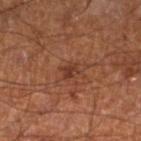Part of a total-body skin-imaging series; this lesion was reviewed on a skin check and was not flagged for biopsy.
A 15 mm close-up tile from a total-body photography series done for melanoma screening.
Automated image analysis of the tile measured a footprint of about 3 mm², a shape eccentricity near 0.7, and a symmetry-axis asymmetry near 0.25. The software also gave border irregularity of about 2.5 on a 0–10 scale, internal color variation of about 1 on a 0–10 scale, and a peripheral color-asymmetry measure near 0.5. The analysis additionally found a classifier nevus-likeness of about 20/100 and a lesion-detection confidence of about 100/100.
The lesion is located on the left lower leg.
A male patient in their 60s.
The recorded lesion diameter is about 2.5 mm.
Captured under cross-polarized illumination.